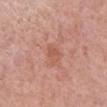The total-body-photography lesion software estimated border irregularity of about 3 on a 0–10 scale and a peripheral color-asymmetry measure near 0.5. And it measured an automated nevus-likeness rating near 0 out of 100 and lesion-presence confidence of about 100/100. From the left forearm. Imaged with white-light lighting. Longest diameter approximately 2.5 mm. A female subject aged approximately 65. Cropped from a total-body skin-imaging series; the visible field is about 15 mm.